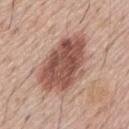The lesion was photographed on a routine skin check and not biopsied; there is no pathology result. A roughly 15 mm field-of-view crop from a total-body skin photograph. On the mid back. A male patient in their mid-50s.